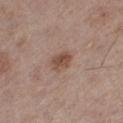Q: Is there a histopathology result?
A: no biopsy performed (imaged during a skin exam)
Q: Lesion location?
A: the left thigh
Q: What kind of image is this?
A: total-body-photography crop, ~15 mm field of view
Q: What are the patient's age and sex?
A: male, aged around 70